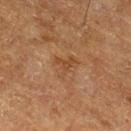• workup — total-body-photography surveillance lesion; no biopsy
• imaging modality — ~15 mm crop, total-body skin-cancer survey
• location — the right thigh
• subject — male, aged 73 to 77
• illumination — cross-polarized